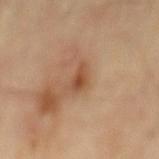Recorded during total-body skin imaging; not selected for excision or biopsy. On the mid back. The subject is a male aged 83 or older. A 15 mm crop from a total-body photograph taken for skin-cancer surveillance. Imaged with cross-polarized lighting. Automated image analysis of the tile measured border irregularity of about 3 on a 0–10 scale, a within-lesion color-variation index near 1.5/10, and peripheral color asymmetry of about 0.5. It also reported an automated nevus-likeness rating near 0 out of 100.